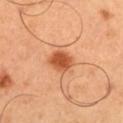Imaged during a routine full-body skin examination; the lesion was not biopsied and no histopathology is available. This is a cross-polarized tile. An algorithmic analysis of the crop reported a border-irregularity rating of about 1.5/10, a within-lesion color-variation index near 2.5/10, and peripheral color asymmetry of about 0.5. A 15 mm crop from a total-body photograph taken for skin-cancer surveillance. Longest diameter approximately 3 mm. The lesion is located on the left thigh. A male subject aged approximately 50.